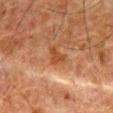notes = total-body-photography surveillance lesion; no biopsy.